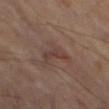  biopsy_status: not biopsied; imaged during a skin examination
  patient:
    sex: male
    age_approx: 70
  lesion_size:
    long_diameter_mm_approx: 3.0
  lighting: cross-polarized
  automated_metrics:
    area_mm2_approx: 3.5
    eccentricity: 0.9
    shape_asymmetry: 0.45
    nevus_likeness_0_100: 0
  site: left thigh
  image:
    source: total-body photography crop
    field_of_view_mm: 15